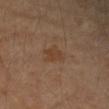No biopsy was performed on this lesion — it was imaged during a full skin examination and was not determined to be concerning. On the arm. A female patient, in their 60s. Automated tile analysis of the lesion measured an outline eccentricity of about 0.55 (0 = round, 1 = elongated) and two-axis asymmetry of about 0.35. It also reported a lesion color around L≈40 a*≈17 b*≈29 in CIELAB, about 5 CIELAB-L* units darker than the surrounding skin, and a normalized border contrast of about 5.5. The analysis additionally found border irregularity of about 3.5 on a 0–10 scale and internal color variation of about 1.5 on a 0–10 scale. The software also gave a nevus-likeness score of about 10/100 and a detector confidence of about 100 out of 100 that the crop contains a lesion. Captured under cross-polarized illumination. About 3 mm across. Cropped from a whole-body photographic skin survey; the tile spans about 15 mm.